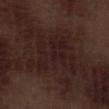Assessment:
Part of a total-body skin-imaging series; this lesion was reviewed on a skin check and was not flagged for biopsy.
Acquisition and patient details:
A lesion tile, about 15 mm wide, cut from a 3D total-body photograph. A male subject, about 70 years old. Automated tile analysis of the lesion measured a mean CIELAB color near L≈19 a*≈16 b*≈15 and a lesion–skin lightness drop of about 4. It also reported a nevus-likeness score of about 0/100. From the right lower leg. This is a white-light tile.